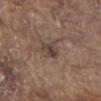  biopsy_status: not biopsied; imaged during a skin examination
  patient:
    sex: male
    age_approx: 80
  image:
    source: total-body photography crop
    field_of_view_mm: 15
  site: front of the torso